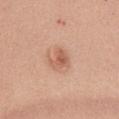Imaged during a routine full-body skin examination; the lesion was not biopsied and no histopathology is available.
The recorded lesion diameter is about 2.5 mm.
The lesion is located on the abdomen.
A female patient about 35 years old.
Imaged with white-light lighting.
An algorithmic analysis of the crop reported a lesion color around L≈59 a*≈24 b*≈32 in CIELAB, about 9 CIELAB-L* units darker than the surrounding skin, and a lesion-to-skin contrast of about 6.5 (normalized; higher = more distinct). The software also gave a lesion-detection confidence of about 100/100.
A 15 mm crop from a total-body photograph taken for skin-cancer surveillance.